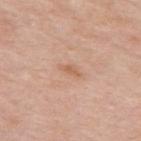workup: catalogued during a skin exam; not biopsied | lighting: white-light | subject: male, approximately 55 years of age | image: ~15 mm crop, total-body skin-cancer survey | location: the upper back | lesion diameter: ≈3 mm.